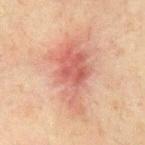biopsy status — total-body-photography surveillance lesion; no biopsy
patient — male, about 65 years old
imaging modality — 15 mm crop, total-body photography
location — the chest